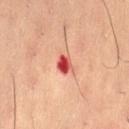No biopsy was performed on this lesion — it was imaged during a full skin examination and was not determined to be concerning.
This image is a 15 mm lesion crop taken from a total-body photograph.
From the right thigh.
Measured at roughly 2.5 mm in maximum diameter.
A male patient, in their mid- to late 50s.